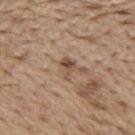Assessment: Recorded during total-body skin imaging; not selected for excision or biopsy. Acquisition and patient details: A 15 mm crop from a total-body photograph taken for skin-cancer surveillance. The patient is a male about 70 years old. Measured at roughly 2.5 mm in maximum diameter. Located on the mid back. Imaged with white-light lighting. An algorithmic analysis of the crop reported an automated nevus-likeness rating near 0 out of 100 and a lesion-detection confidence of about 100/100.